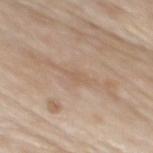– follow-up · imaged on a skin check; not biopsied
– patient · male, about 85 years old or older
– location · the upper back
– illumination · white-light illumination
– imaging modality · ~15 mm tile from a whole-body skin photo